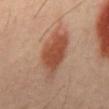Notes:
- workup — imaged on a skin check; not biopsied
- anatomic site — the mid back
- illumination — cross-polarized illumination
- subject — male, approximately 45 years of age
- imaging modality — ~15 mm crop, total-body skin-cancer survey
- automated metrics — a footprint of about 15 mm², a shape eccentricity near 0.85, and a symmetry-axis asymmetry near 0.3; internal color variation of about 3.5 on a 0–10 scale and a peripheral color-asymmetry measure near 1
- size — ≈6 mm